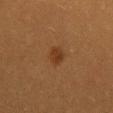<case>
<lesion_size>
  <long_diameter_mm_approx>2.5</long_diameter_mm_approx>
</lesion_size>
<image>
  <source>total-body photography crop</source>
  <field_of_view_mm>15</field_of_view_mm>
</image>
<automated_metrics>
  <cielab_L>29</cielab_L>
  <cielab_a>18</cielab_a>
  <cielab_b>29</cielab_b>
  <vs_skin_contrast_norm>7.0</vs_skin_contrast_norm>
  <border_irregularity_0_10>1.5</border_irregularity_0_10>
  <color_variation_0_10>1.5</color_variation_0_10>
  <peripheral_color_asymmetry>0.5</peripheral_color_asymmetry>
</automated_metrics>
<lighting>cross-polarized</lighting>
<site>mid back</site>
<patient>
  <sex>female</sex>
  <age_approx>40</age_approx>
</patient>
</case>